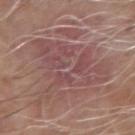The lesion was photographed on a routine skin check and not biopsied; there is no pathology result. The lesion's longest dimension is about 11 mm. Cropped from a total-body skin-imaging series; the visible field is about 15 mm. Located on the left forearm. Imaged with white-light lighting. A male patient roughly 65 years of age.